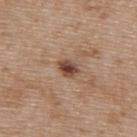Imaged during a routine full-body skin examination; the lesion was not biopsied and no histopathology is available.